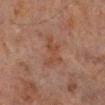Impression: This lesion was catalogued during total-body skin photography and was not selected for biopsy. Context: A male subject aged 63–67. This image is a 15 mm lesion crop taken from a total-body photograph. Located on the left lower leg. The tile uses cross-polarized illumination.